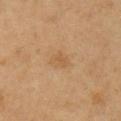Clinical impression:
Recorded during total-body skin imaging; not selected for excision or biopsy.
Background:
A male patient aged approximately 60. This image is a 15 mm lesion crop taken from a total-body photograph. The lesion's longest dimension is about 2.5 mm. Automated image analysis of the tile measured a footprint of about 4 mm². It also reported a mean CIELAB color near L≈51 a*≈17 b*≈34, roughly 5 lightness units darker than nearby skin, and a lesion-to-skin contrast of about 4.5 (normalized; higher = more distinct). It also reported border irregularity of about 2 on a 0–10 scale, a color-variation rating of about 1.5/10, and peripheral color asymmetry of about 0.5. On the right upper arm. Captured under cross-polarized illumination.